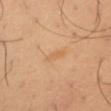Assessment:
The lesion was tiled from a total-body skin photograph and was not biopsied.
Clinical summary:
A close-up tile cropped from a whole-body skin photograph, about 15 mm across. Captured under cross-polarized illumination. The lesion-visualizer software estimated a footprint of about 2.5 mm², an eccentricity of roughly 0.95, and two-axis asymmetry of about 0.35. The software also gave an average lesion color of about L≈62 a*≈20 b*≈39 (CIELAB) and a normalized lesion–skin contrast near 4.5. The software also gave a classifier nevus-likeness of about 0/100 and a lesion-detection confidence of about 100/100. The lesion is located on the right thigh. The patient is a male approximately 50 years of age. Approximately 3 mm at its widest.